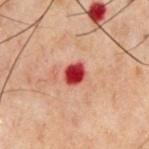imaging modality: ~15 mm crop, total-body skin-cancer survey; anatomic site: the chest; patient: male, approximately 60 years of age; illumination: cross-polarized.